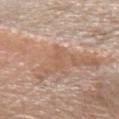Acquisition and patient details:
A female patient approximately 60 years of age. Cropped from a total-body skin-imaging series; the visible field is about 15 mm. The lesion-visualizer software estimated a border-irregularity rating of about 5/10, internal color variation of about 0 on a 0–10 scale, and peripheral color asymmetry of about 0. Imaged with white-light lighting. The lesion is on the left forearm.